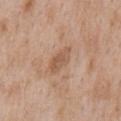Clinical impression:
The lesion was tiled from a total-body skin photograph and was not biopsied.
Context:
A male subject, aged 63 to 67. A region of skin cropped from a whole-body photographic capture, roughly 15 mm wide. The lesion is on the chest.